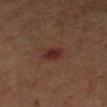Notes:
- biopsy status · catalogued during a skin exam; not biopsied
- illumination · cross-polarized illumination
- automated metrics · a footprint of about 4.5 mm², an eccentricity of roughly 0.65, and a symmetry-axis asymmetry near 0.2; a mean CIELAB color near L≈26 a*≈21 b*≈20, a lesion–skin lightness drop of about 7, and a normalized border contrast of about 8.5; a border-irregularity index near 2/10, a color-variation rating of about 2/10, and peripheral color asymmetry of about 0.5; a nevus-likeness score of about 10/100
- diameter · ~2.5 mm (longest diameter)
- acquisition · ~15 mm crop, total-body skin-cancer survey
- subject · male, aged approximately 60
- site · the right lower leg